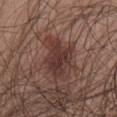Captured during whole-body skin photography for melanoma surveillance; the lesion was not biopsied. From the abdomen. The patient is a male aged 28–32. A 15 mm close-up extracted from a 3D total-body photography capture.